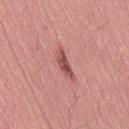Clinical impression:
Imaged during a routine full-body skin examination; the lesion was not biopsied and no histopathology is available.
Clinical summary:
The lesion-visualizer software estimated a lesion area of about 4.5 mm², a shape eccentricity near 0.95, and a symmetry-axis asymmetry near 0.3. A male subject, approximately 30 years of age. A region of skin cropped from a whole-body photographic capture, roughly 15 mm wide. About 4 mm across. Located on the right thigh. Captured under white-light illumination.